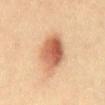No biopsy was performed on this lesion — it was imaged during a full skin examination and was not determined to be concerning.
This is a cross-polarized tile.
The patient is a male aged 38 to 42.
A 15 mm close-up tile from a total-body photography series done for melanoma screening.
Measured at roughly 5 mm in maximum diameter.
The lesion is on the abdomen.